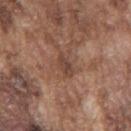Context: Measured at roughly 3 mm in maximum diameter. A male patient about 75 years old. A region of skin cropped from a whole-body photographic capture, roughly 15 mm wide. The lesion is located on the mid back.